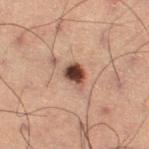Q: Is there a histopathology result?
A: catalogued during a skin exam; not biopsied
Q: Who is the patient?
A: male, in their 60s
Q: What kind of image is this?
A: total-body-photography crop, ~15 mm field of view